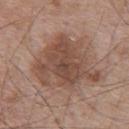Captured during whole-body skin photography for melanoma surveillance; the lesion was not biopsied. The lesion is on the upper back. A 15 mm crop from a total-body photograph taken for skin-cancer surveillance. This is a white-light tile. A male patient aged approximately 65.